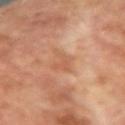Findings:
* notes — no biopsy performed (imaged during a skin exam)